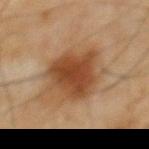Q: Is there a histopathology result?
A: imaged on a skin check; not biopsied
Q: Patient demographics?
A: male, aged 63–67
Q: What did automated image analysis measure?
A: a shape eccentricity near 0.7 and a shape-asymmetry score of about 0.25 (0 = symmetric); a mean CIELAB color near L≈38 a*≈20 b*≈31 and a normalized border contrast of about 9.5
Q: What is the lesion's diameter?
A: ≈6 mm
Q: What is the imaging modality?
A: 15 mm crop, total-body photography
Q: Where on the body is the lesion?
A: the chest